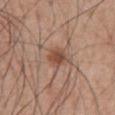Impression: The lesion was photographed on a routine skin check and not biopsied; there is no pathology result. Image and clinical context: A lesion tile, about 15 mm wide, cut from a 3D total-body photograph. From the abdomen. A male subject aged 53–57. Automated tile analysis of the lesion measured border irregularity of about 2 on a 0–10 scale, a color-variation rating of about 4/10, and radial color variation of about 1. About 3 mm across. This is a white-light tile.